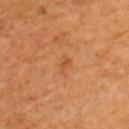follow-up: total-body-photography surveillance lesion; no biopsy | image-analysis metrics: a shape eccentricity near 0.8 and two-axis asymmetry of about 0.35; a mean CIELAB color near L≈52 a*≈25 b*≈41, roughly 6 lightness units darker than nearby skin, and a normalized border contrast of about 4.5; a nevus-likeness score of about 0/100 and a lesion-detection confidence of about 100/100 | imaging modality: total-body-photography crop, ~15 mm field of view | subject: female, aged 48 to 52 | illumination: cross-polarized illumination | location: the left upper arm | diameter: ≈2.5 mm.